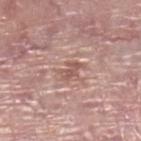{
  "biopsy_status": "not biopsied; imaged during a skin examination",
  "image": {
    "source": "total-body photography crop",
    "field_of_view_mm": 15
  },
  "site": "right lower leg",
  "patient": {
    "sex": "female",
    "age_approx": 60
  }
}